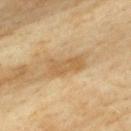No biopsy was performed on this lesion — it was imaged during a full skin examination and was not determined to be concerning. The total-body-photography lesion software estimated an average lesion color of about L≈54 a*≈15 b*≈37 (CIELAB), roughly 9 lightness units darker than nearby skin, and a normalized border contrast of about 6.5. The analysis additionally found an automated nevus-likeness rating near 0 out of 100. The lesion's longest dimension is about 4.5 mm. The tile uses cross-polarized illumination. The lesion is on the upper back. A female subject, about 60 years old. A 15 mm close-up tile from a total-body photography series done for melanoma screening.